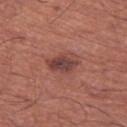* lesion diameter · ≈4 mm
* lighting · white-light illumination
* subject · male, aged 63–67
* image source · ~15 mm crop, total-body skin-cancer survey
* body site · the left thigh